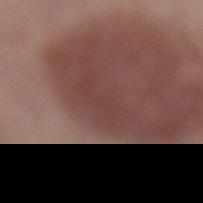{
  "site": "left lower leg",
  "automated_metrics": {
    "area_mm2_approx": 8.0,
    "eccentricity": 0.9,
    "shape_asymmetry": 0.3,
    "cielab_L": 40,
    "cielab_a": 20,
    "cielab_b": 21,
    "vs_skin_darker_L": 4.0,
    "vs_skin_contrast_norm": 3.5,
    "nevus_likeness_0_100": 0,
    "lesion_detection_confidence_0_100": 25
  },
  "lighting": "white-light",
  "patient": {
    "sex": "male",
    "age_approx": 70
  },
  "image": {
    "source": "total-body photography crop",
    "field_of_view_mm": 15
  },
  "lesion_size": {
    "long_diameter_mm_approx": 4.5
  }
}